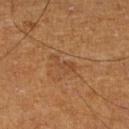  biopsy_status: not biopsied; imaged during a skin examination
  image:
    source: total-body photography crop
    field_of_view_mm: 15
  lesion_size:
    long_diameter_mm_approx: 3.5
  lighting: cross-polarized
  patient:
    sex: male
    age_approx: 60
  automated_metrics:
    border_irregularity_0_10: 6.5
    color_variation_0_10: 0.5
    peripheral_color_asymmetry: 0.0
  site: right lower leg